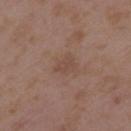Q: Was this lesion biopsied?
A: total-body-photography surveillance lesion; no biopsy
Q: What is the imaging modality?
A: 15 mm crop, total-body photography
Q: How was the tile lit?
A: white-light illumination
Q: Lesion size?
A: ≈2.5 mm
Q: Where on the body is the lesion?
A: the left upper arm
Q: Who is the patient?
A: female, roughly 35 years of age